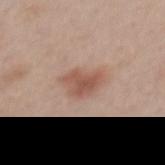Impression: Imaged during a routine full-body skin examination; the lesion was not biopsied and no histopathology is available. Context: The tile uses white-light illumination. The lesion's longest dimension is about 4 mm. Cropped from a whole-body photographic skin survey; the tile spans about 15 mm. The lesion is on the mid back. A female patient aged 38–42.